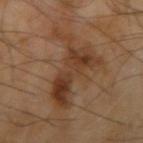The lesion was tiled from a total-body skin photograph and was not biopsied.